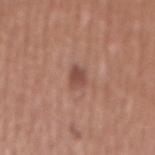notes = total-body-photography surveillance lesion; no biopsy
illumination = white-light
diameter = about 2.5 mm
body site = the abdomen
image = total-body-photography crop, ~15 mm field of view
automated metrics = a mean CIELAB color near L≈48 a*≈22 b*≈26, about 10 CIELAB-L* units darker than the surrounding skin, and a normalized border contrast of about 7.5; a classifier nevus-likeness of about 75/100
patient = male, aged approximately 45